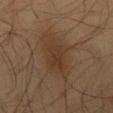Findings:
- biopsy status · total-body-photography surveillance lesion; no biopsy
- site · the mid back
- tile lighting · cross-polarized illumination
- image source · 15 mm crop, total-body photography
- size · ≈7.5 mm
- subject · male, about 55 years old